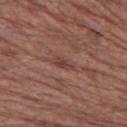Part of a total-body skin-imaging series; this lesion was reviewed on a skin check and was not flagged for biopsy.
A male subject about 75 years old.
A roughly 15 mm field-of-view crop from a total-body skin photograph.
Captured under white-light illumination.
Measured at roughly 2.5 mm in maximum diameter.
From the left thigh.